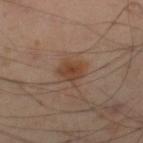Clinical impression:
The lesion was tiled from a total-body skin photograph and was not biopsied.
Acquisition and patient details:
A male subject in their mid-50s. The total-body-photography lesion software estimated a footprint of about 6 mm² and an outline eccentricity of about 0.55 (0 = round, 1 = elongated). This image is a 15 mm lesion crop taken from a total-body photograph. Measured at roughly 3 mm in maximum diameter. From the leg.